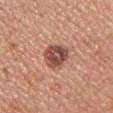Acquisition and patient details: Automated tile analysis of the lesion measured a shape eccentricity near 0.55 and a symmetry-axis asymmetry near 0.2. It also reported a lesion color around L≈50 a*≈24 b*≈27 in CIELAB, about 14 CIELAB-L* units darker than the surrounding skin, and a normalized lesion–skin contrast near 10. The recorded lesion diameter is about 4 mm. The lesion is on the left upper arm. A roughly 15 mm field-of-view crop from a total-body skin photograph. A female subject, aged 33–37. The tile uses white-light illumination.